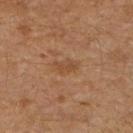The lesion was tiled from a total-body skin photograph and was not biopsied. The tile uses cross-polarized illumination. The lesion is located on the left leg. The patient is a male roughly 30 years of age. An algorithmic analysis of the crop reported a lesion color around L≈46 a*≈19 b*≈33 in CIELAB and a normalized lesion–skin contrast near 5. The lesion's longest dimension is about 3 mm. This image is a 15 mm lesion crop taken from a total-body photograph.